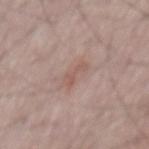{
  "biopsy_status": "not biopsied; imaged during a skin examination",
  "lesion_size": {
    "long_diameter_mm_approx": 3.0
  },
  "patient": {
    "sex": "male",
    "age_approx": 65
  },
  "site": "mid back",
  "image": {
    "source": "total-body photography crop",
    "field_of_view_mm": 15
  }
}